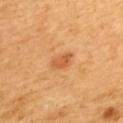Recorded during total-body skin imaging; not selected for excision or biopsy. This image is a 15 mm lesion crop taken from a total-body photograph. Located on the upper back. A female patient approximately 55 years of age.